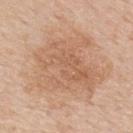Imaged during a routine full-body skin examination; the lesion was not biopsied and no histopathology is available.